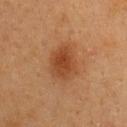Assessment: Recorded during total-body skin imaging; not selected for excision or biopsy. Image and clinical context: A close-up tile cropped from a whole-body skin photograph, about 15 mm across. On the upper back. A female patient approximately 40 years of age. Imaged with cross-polarized lighting. Measured at roughly 4 mm in maximum diameter.